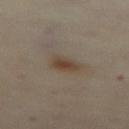{"biopsy_status": "not biopsied; imaged during a skin examination", "patient": {"sex": "female", "age_approx": 60}, "site": "abdomen", "image": {"source": "total-body photography crop", "field_of_view_mm": 15}}